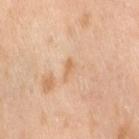Impression:
The lesion was photographed on a routine skin check and not biopsied; there is no pathology result.
Acquisition and patient details:
Located on the right thigh. A female subject, roughly 55 years of age. This image is a 15 mm lesion crop taken from a total-body photograph.